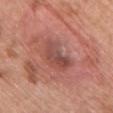follow-up: no biopsy performed (imaged during a skin exam)
image: 15 mm crop, total-body photography
anatomic site: the chest
subject: female, aged around 60
illumination: white-light
TBP lesion metrics: a mean CIELAB color near L≈48 a*≈25 b*≈26 and a normalized border contrast of about 6.5; a border-irregularity index near 4/10 and a peripheral color-asymmetry measure near 2; an automated nevus-likeness rating near 0 out of 100 and a lesion-detection confidence of about 100/100
lesion diameter: ≈4.5 mm